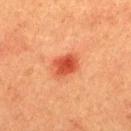No biopsy was performed on this lesion — it was imaged during a full skin examination and was not determined to be concerning. An algorithmic analysis of the crop reported a symmetry-axis asymmetry near 0.2. It also reported lesion-presence confidence of about 100/100. Cropped from a whole-body photographic skin survey; the tile spans about 15 mm. Captured under cross-polarized illumination. Approximately 3.5 mm at its widest. On the upper back. A male patient aged 38 to 42.